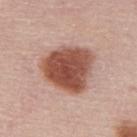The lesion was photographed on a routine skin check and not biopsied; there is no pathology result. The total-body-photography lesion software estimated a footprint of about 24 mm², an outline eccentricity of about 0.55 (0 = round, 1 = elongated), and a symmetry-axis asymmetry near 0.25. The analysis additionally found a lesion color around L≈51 a*≈24 b*≈28 in CIELAB and roughly 17 lightness units darker than nearby skin. The tile uses white-light illumination. A male patient, aged 58–62. On the mid back. About 6 mm across. Cropped from a total-body skin-imaging series; the visible field is about 15 mm.